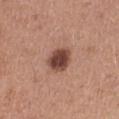This is a white-light tile.
A 15 mm close-up tile from a total-body photography series done for melanoma screening.
The subject is a female in their 40s.
The lesion is located on the right thigh.